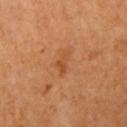Assessment:
Captured during whole-body skin photography for melanoma surveillance; the lesion was not biopsied.
Acquisition and patient details:
A female patient, in their mid- to late 50s. The lesion is on the right upper arm. The lesion's longest dimension is about 3 mm. Imaged with cross-polarized lighting. This image is a 15 mm lesion crop taken from a total-body photograph. An algorithmic analysis of the crop reported a lesion area of about 3.5 mm², an outline eccentricity of about 0.9 (0 = round, 1 = elongated), and two-axis asymmetry of about 0.4. The software also gave an average lesion color of about L≈51 a*≈27 b*≈40 (CIELAB) and a normalized lesion–skin contrast near 5.5. The software also gave a detector confidence of about 100 out of 100 that the crop contains a lesion.